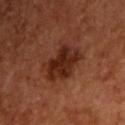Q: Was a biopsy performed?
A: catalogued during a skin exam; not biopsied
Q: How large is the lesion?
A: about 4.5 mm
Q: What kind of image is this?
A: ~15 mm crop, total-body skin-cancer survey
Q: Illumination type?
A: cross-polarized illumination
Q: Where on the body is the lesion?
A: the chest
Q: What did automated image analysis measure?
A: an automated nevus-likeness rating near 70 out of 100 and a detector confidence of about 100 out of 100 that the crop contains a lesion
Q: Patient demographics?
A: male, approximately 55 years of age